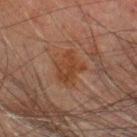Q: Was this lesion biopsied?
A: imaged on a skin check; not biopsied
Q: Patient demographics?
A: male, about 65 years old
Q: How large is the lesion?
A: ≈4 mm
Q: What did automated image analysis measure?
A: a lesion color around L≈33 a*≈18 b*≈27 in CIELAB, a lesion–skin lightness drop of about 5, and a lesion-to-skin contrast of about 7 (normalized; higher = more distinct); a border-irregularity index near 4/10, internal color variation of about 2.5 on a 0–10 scale, and a peripheral color-asymmetry measure near 1
Q: What is the anatomic site?
A: the head or neck
Q: What kind of image is this?
A: 15 mm crop, total-body photography
Q: What lighting was used for the tile?
A: cross-polarized